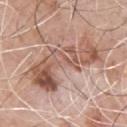biopsy status: catalogued during a skin exam; not biopsied
tile lighting: white-light
subject: male, aged 68–72
site: the front of the torso
automated metrics: an eccentricity of roughly 0.9; a nevus-likeness score of about 30/100 and a detector confidence of about 100 out of 100 that the crop contains a lesion
image: 15 mm crop, total-body photography